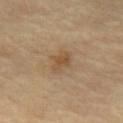follow-up: imaged on a skin check; not biopsied
lighting: cross-polarized
lesion diameter: ~3 mm (longest diameter)
site: the abdomen
automated lesion analysis: an outline eccentricity of about 0.8 (0 = round, 1 = elongated) and two-axis asymmetry of about 0.35; a border-irregularity index near 4/10, internal color variation of about 1.5 on a 0–10 scale, and peripheral color asymmetry of about 0.5
patient: male, approximately 70 years of age
image: 15 mm crop, total-body photography